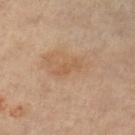Captured during whole-body skin photography for melanoma surveillance; the lesion was not biopsied. Longest diameter approximately 3.5 mm. The lesion is on the right thigh. A female patient aged around 75. A lesion tile, about 15 mm wide, cut from a 3D total-body photograph.